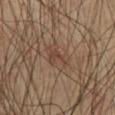notes = catalogued during a skin exam; not biopsied | diameter = ≈4 mm | image source = ~15 mm tile from a whole-body skin photo | location = the front of the torso | patient = male, roughly 60 years of age | image-analysis metrics = an area of roughly 7.5 mm², an eccentricity of roughly 0.8, and a symmetry-axis asymmetry near 0.35; a lesion color around L≈43 a*≈17 b*≈26 in CIELAB and about 6 CIELAB-L* units darker than the surrounding skin.